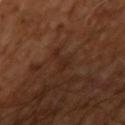biopsy status: total-body-photography surveillance lesion; no biopsy
size: about 3.5 mm
lighting: cross-polarized illumination
location: the arm
image-analysis metrics: an area of roughly 3 mm², a shape eccentricity near 0.9, and a shape-asymmetry score of about 0.7 (0 = symmetric); a classifier nevus-likeness of about 0/100 and lesion-presence confidence of about 90/100
patient: in their mid- to late 60s
image: total-body-photography crop, ~15 mm field of view